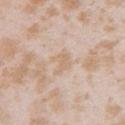| field | value |
|---|---|
| follow-up | imaged on a skin check; not biopsied |
| location | the arm |
| acquisition | total-body-photography crop, ~15 mm field of view |
| lesion size | ~3 mm (longest diameter) |
| illumination | white-light illumination |
| subject | female, aged 23 to 27 |